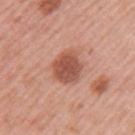The lesion was tiled from a total-body skin photograph and was not biopsied.
Approximately 3.5 mm at its widest.
From the left upper arm.
Captured under white-light illumination.
A female subject roughly 40 years of age.
Cropped from a whole-body photographic skin survey; the tile spans about 15 mm.
Automated image analysis of the tile measured an average lesion color of about L≈53 a*≈26 b*≈30 (CIELAB) and roughly 13 lightness units darker than nearby skin.